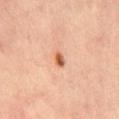follow-up: no biopsy performed (imaged during a skin exam) | lighting: cross-polarized illumination | image source: ~15 mm crop, total-body skin-cancer survey | diameter: ~2 mm (longest diameter) | body site: the mid back | patient: male, about 65 years old | TBP lesion metrics: a footprint of about 2 mm², an outline eccentricity of about 0.8 (0 = round, 1 = elongated), and a shape-asymmetry score of about 0.2 (0 = symmetric); a border-irregularity index near 1.5/10, internal color variation of about 2 on a 0–10 scale, and a peripheral color-asymmetry measure near 0.5; a classifier nevus-likeness of about 100/100 and lesion-presence confidence of about 100/100.